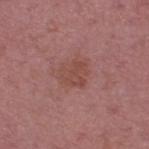workup: catalogued during a skin exam; not biopsied | size: ~3 mm (longest diameter) | illumination: white-light | site: the upper back | image: ~15 mm tile from a whole-body skin photo | subject: male, aged approximately 55 | automated lesion analysis: a mean CIELAB color near L≈47 a*≈24 b*≈24, about 6 CIELAB-L* units darker than the surrounding skin, and a lesion-to-skin contrast of about 5.5 (normalized; higher = more distinct); a border-irregularity rating of about 3/10, internal color variation of about 1.5 on a 0–10 scale, and peripheral color asymmetry of about 0.5; a nevus-likeness score of about 0/100.